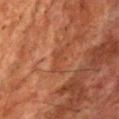notes — catalogued during a skin exam; not biopsied
site — the chest
image — total-body-photography crop, ~15 mm field of view
illumination — cross-polarized
automated metrics — an average lesion color of about L≈36 a*≈23 b*≈29 (CIELAB) and about 5 CIELAB-L* units darker than the surrounding skin; a lesion-detection confidence of about 80/100
diameter — ≈4 mm
patient — male, approximately 65 years of age